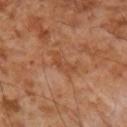Background:
Located on the arm. The subject is a male aged around 55. A roughly 15 mm field-of-view crop from a total-body skin photograph. Automated image analysis of the tile measured a footprint of about 5 mm² and an eccentricity of roughly 0.95. The software also gave a mean CIELAB color near L≈48 a*≈24 b*≈35 and about 7 CIELAB-L* units darker than the surrounding skin. It also reported an automated nevus-likeness rating near 0 out of 100 and a detector confidence of about 75 out of 100 that the crop contains a lesion.